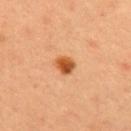Imaged during a routine full-body skin examination; the lesion was not biopsied and no histopathology is available. A 15 mm close-up tile from a total-body photography series done for melanoma screening. Longest diameter approximately 2.5 mm. Located on the upper back. The tile uses cross-polarized illumination. A male patient, approximately 35 years of age.